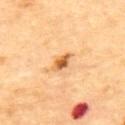No biopsy was performed on this lesion — it was imaged during a full skin examination and was not determined to be concerning. On the upper back. Approximately 2.5 mm at its widest. A male patient in their mid- to late 80s or older. A region of skin cropped from a whole-body photographic capture, roughly 15 mm wide.